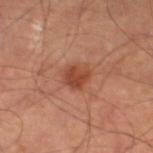Impression: Recorded during total-body skin imaging; not selected for excision or biopsy.